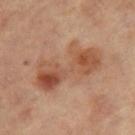Assessment: Part of a total-body skin-imaging series; this lesion was reviewed on a skin check and was not flagged for biopsy. Background: The lesion is on the left leg. The recorded lesion diameter is about 8 mm. Captured under cross-polarized illumination. A 15 mm close-up extracted from a 3D total-body photography capture. A female subject aged around 65.